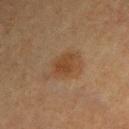{
  "biopsy_status": "not biopsied; imaged during a skin examination",
  "lesion_size": {
    "long_diameter_mm_approx": 2.5
  },
  "patient": {
    "sex": "male",
    "age_approx": 60
  },
  "lighting": "cross-polarized",
  "site": "upper back",
  "image": {
    "source": "total-body photography crop",
    "field_of_view_mm": 15
  }
}